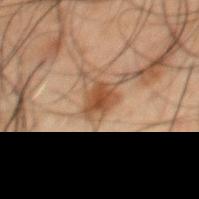Q: Is there a histopathology result?
A: catalogued during a skin exam; not biopsied
Q: What are the patient's age and sex?
A: male, about 50 years old
Q: What is the imaging modality?
A: total-body-photography crop, ~15 mm field of view
Q: Illumination type?
A: cross-polarized
Q: Where on the body is the lesion?
A: the mid back